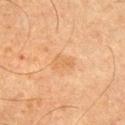| feature | finding |
|---|---|
| biopsy status | imaged on a skin check; not biopsied |